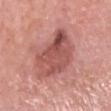Assessment:
No biopsy was performed on this lesion — it was imaged during a full skin examination and was not determined to be concerning.
Acquisition and patient details:
Measured at roughly 7 mm in maximum diameter. The patient is a male aged 83 or over. The tile uses white-light illumination. An algorithmic analysis of the crop reported a lesion area of about 23 mm², an eccentricity of roughly 0.8, and a symmetry-axis asymmetry near 0.25. The analysis additionally found a border-irregularity rating of about 3.5/10 and a within-lesion color-variation index near 7/10. Located on the head or neck. A 15 mm crop from a total-body photograph taken for skin-cancer surveillance.